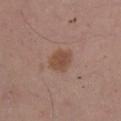• body site: the right lower leg
• acquisition: ~15 mm tile from a whole-body skin photo
• illumination: white-light
• size: ~3 mm (longest diameter)
• subject: male, aged 53–57